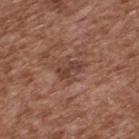biopsy_status: not biopsied; imaged during a skin examination